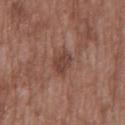The lesion was tiled from a total-body skin photograph and was not biopsied. The tile uses white-light illumination. The subject is a male approximately 50 years of age. From the mid back. A 15 mm crop from a total-body photograph taken for skin-cancer surveillance. The recorded lesion diameter is about 2.5 mm. The lesion-visualizer software estimated a within-lesion color-variation index near 2.5/10 and radial color variation of about 1.